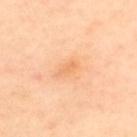Q: Was this lesion biopsied?
A: total-body-photography surveillance lesion; no biopsy
Q: What lighting was used for the tile?
A: cross-polarized illumination
Q: Patient demographics?
A: female, in their mid-50s
Q: What kind of image is this?
A: 15 mm crop, total-body photography
Q: What did automated image analysis measure?
A: a lesion area of about 2.5 mm² and an eccentricity of roughly 0.9; a mean CIELAB color near L≈74 a*≈26 b*≈44, a lesion–skin lightness drop of about 7, and a normalized lesion–skin contrast near 5; a border-irregularity index near 3.5/10 and internal color variation of about 0 on a 0–10 scale
Q: Where on the body is the lesion?
A: the upper back